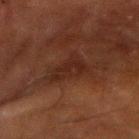Clinical impression:
Captured during whole-body skin photography for melanoma surveillance; the lesion was not biopsied.
Acquisition and patient details:
The lesion is located on the arm. The tile uses cross-polarized illumination. The patient is a male roughly 65 years of age. The recorded lesion diameter is about 4 mm. Automated tile analysis of the lesion measured a lesion color around L≈20 a*≈18 b*≈21 in CIELAB, roughly 5 lightness units darker than nearby skin, and a normalized lesion–skin contrast near 6. The analysis additionally found a border-irregularity index near 5/10, a within-lesion color-variation index near 2.5/10, and radial color variation of about 1. A 15 mm close-up extracted from a 3D total-body photography capture.